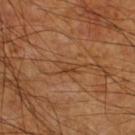• biopsy status: no biopsy performed (imaged during a skin exam)
• diameter: ~2.5 mm (longest diameter)
• site: the right lower leg
• tile lighting: cross-polarized
• patient: male, aged approximately 60
• image: 15 mm crop, total-body photography